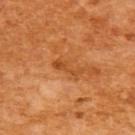follow-up=no biopsy performed (imaged during a skin exam) | image=~15 mm crop, total-body skin-cancer survey | lesion size=about 4 mm | location=the back | automated metrics=a classifier nevus-likeness of about 0/100 and a lesion-detection confidence of about 100/100 | subject=female, in their mid-50s | tile lighting=cross-polarized illumination.